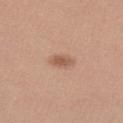follow-up = imaged on a skin check; not biopsied
diameter = ≈2.5 mm
automated metrics = a lesion color around L≈56 a*≈22 b*≈30 in CIELAB, roughly 10 lightness units darker than nearby skin, and a normalized lesion–skin contrast near 6.5
patient = female, approximately 30 years of age
image source = ~15 mm crop, total-body skin-cancer survey
body site = the left forearm